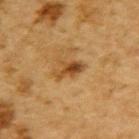Part of a total-body skin-imaging series; this lesion was reviewed on a skin check and was not flagged for biopsy. From the upper back. The tile uses cross-polarized illumination. Cropped from a total-body skin-imaging series; the visible field is about 15 mm. Longest diameter approximately 3.5 mm. The patient is a male about 85 years old or older.